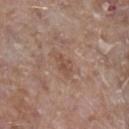<record>
<biopsy_status>not biopsied; imaged during a skin examination</biopsy_status>
<site>right lower leg</site>
<image>
  <source>total-body photography crop</source>
  <field_of_view_mm>15</field_of_view_mm>
</image>
<lesion_size>
  <long_diameter_mm_approx>3.0</long_diameter_mm_approx>
</lesion_size>
<automated_metrics>
  <area_mm2_approx>4.0</area_mm2_approx>
  <eccentricity>0.8</eccentricity>
  <shape_asymmetry>0.35</shape_asymmetry>
</automated_metrics>
<lighting>white-light</lighting>
<patient>
  <sex>male</sex>
  <age_approx>45</age_approx>
</patient>
</record>